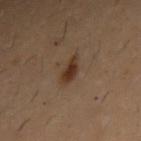The lesion was photographed on a routine skin check and not biopsied; there is no pathology result.
Longest diameter approximately 3 mm.
A region of skin cropped from a whole-body photographic capture, roughly 15 mm wide.
The subject is a male about 30 years old.
An algorithmic analysis of the crop reported a footprint of about 4.5 mm², an outline eccentricity of about 0.85 (0 = round, 1 = elongated), and a shape-asymmetry score of about 0.2 (0 = symmetric). And it measured a lesion–skin lightness drop of about 8. The analysis additionally found border irregularity of about 2 on a 0–10 scale and internal color variation of about 3 on a 0–10 scale.
From the right upper arm.
The tile uses cross-polarized illumination.